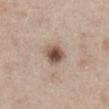The lesion was tiled from a total-body skin photograph and was not biopsied. Approximately 3 mm at its widest. A lesion tile, about 15 mm wide, cut from a 3D total-body photograph. A female subject, in their 40s. From the mid back. Captured under white-light illumination.